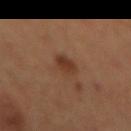notes = imaged on a skin check; not biopsied | acquisition = total-body-photography crop, ~15 mm field of view | subject = female | lesion size = about 3.5 mm | lighting = cross-polarized illumination | site = the back.